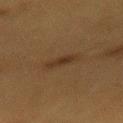Impression:
No biopsy was performed on this lesion — it was imaged during a full skin examination and was not determined to be concerning.
Clinical summary:
The lesion is on the mid back. An algorithmic analysis of the crop reported a footprint of about 3 mm². The software also gave a lesion color around L≈26 a*≈14 b*≈25 in CIELAB, about 6 CIELAB-L* units darker than the surrounding skin, and a normalized lesion–skin contrast near 7. This is a cross-polarized tile. A 15 mm crop from a total-body photograph taken for skin-cancer surveillance. A female subject aged 38 to 42. The lesion's longest dimension is about 2.5 mm.